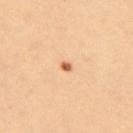  biopsy_status: not biopsied; imaged during a skin examination
  patient:
    sex: female
    age_approx: 40
  site: upper back
  lighting: cross-polarized
  image:
    source: total-body photography crop
    field_of_view_mm: 15
  lesion_size:
    long_diameter_mm_approx: 1.5